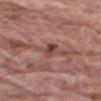<tbp_lesion>
  <biopsy_status>not biopsied; imaged during a skin examination</biopsy_status>
  <lighting>white-light</lighting>
  <automated_metrics>
    <eccentricity>0.8</eccentricity>
    <shape_asymmetry>0.2</shape_asymmetry>
    <border_irregularity_0_10>2.0</border_irregularity_0_10>
    <peripheral_color_asymmetry>2.0</peripheral_color_asymmetry>
    <nevus_likeness_0_100>0</nevus_likeness_0_100>
  </automated_metrics>
  <image>
    <source>total-body photography crop</source>
    <field_of_view_mm>15</field_of_view_mm>
  </image>
  <patient>
    <sex>male</sex>
    <age_approx>70</age_approx>
  </patient>
  <site>right upper arm</site>
</tbp_lesion>